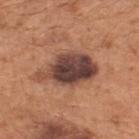biopsy_status: not biopsied; imaged during a skin examination
patient:
  sex: male
  age_approx: 65
lesion_size:
  long_diameter_mm_approx: 7.5
lighting: white-light
image:
  source: total-body photography crop
  field_of_view_mm: 15
site: upper back
automated_metrics:
  cielab_L: 43
  cielab_a: 21
  cielab_b: 25
  vs_skin_darker_L: 16.0
  border_irregularity_0_10: 3.5
  color_variation_0_10: 7.5
  peripheral_color_asymmetry: 2.5
  nevus_likeness_0_100: 45
  lesion_detection_confidence_0_100: 100